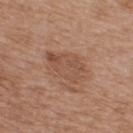Recorded during total-body skin imaging; not selected for excision or biopsy. The subject is a male approximately 65 years of age. This image is a 15 mm lesion crop taken from a total-body photograph. Imaged with white-light lighting. The recorded lesion diameter is about 4.5 mm. On the upper back.